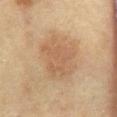workup: imaged on a skin check; not biopsied | acquisition: total-body-photography crop, ~15 mm field of view | tile lighting: cross-polarized illumination | patient: female, roughly 60 years of age | image-analysis metrics: an area of roughly 16 mm², an outline eccentricity of about 0.7 (0 = round, 1 = elongated), and two-axis asymmetry of about 0.3; an automated nevus-likeness rating near 10 out of 100 and lesion-presence confidence of about 100/100 | site: the lower back | size: about 5.5 mm.